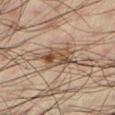The lesion was tiled from a total-body skin photograph and was not biopsied. Captured under cross-polarized illumination. From the left lower leg. A roughly 15 mm field-of-view crop from a total-body skin photograph. The subject is a male roughly 35 years of age. Automated tile analysis of the lesion measured a footprint of about 8.5 mm², an eccentricity of roughly 0.85, and two-axis asymmetry of about 0.25. It also reported a classifier nevus-likeness of about 60/100 and a detector confidence of about 100 out of 100 that the crop contains a lesion.